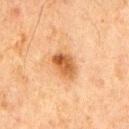| feature | finding |
|---|---|
| biopsy status | catalogued during a skin exam; not biopsied |